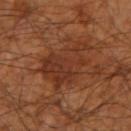Imaged during a routine full-body skin examination; the lesion was not biopsied and no histopathology is available. The total-body-photography lesion software estimated a footprint of about 21 mm², an outline eccentricity of about 0.75 (0 = round, 1 = elongated), and two-axis asymmetry of about 0.4. And it measured border irregularity of about 6 on a 0–10 scale, internal color variation of about 3.5 on a 0–10 scale, and radial color variation of about 1. The analysis additionally found an automated nevus-likeness rating near 5 out of 100. Longest diameter approximately 7 mm. The tile uses cross-polarized illumination. The subject is a male approximately 60 years of age. A 15 mm crop from a total-body photograph taken for skin-cancer surveillance. The lesion is located on the right forearm.